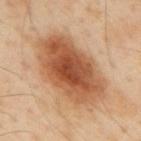Cropped from a whole-body photographic skin survey; the tile spans about 15 mm. The patient is a male aged 53 to 57. The lesion is located on the mid back.